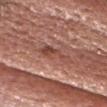This lesion was catalogued during total-body skin photography and was not selected for biopsy. A male subject roughly 65 years of age. On the head or neck. Cropped from a total-body skin-imaging series; the visible field is about 15 mm. About 4.5 mm across.